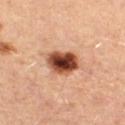{"biopsy_status": "not biopsied; imaged during a skin examination", "site": "right thigh", "image": {"source": "total-body photography crop", "field_of_view_mm": 15}, "patient": {"sex": "female", "age_approx": 45}}